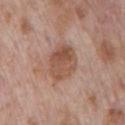Findings:
* lighting · white-light
* subject · male, aged around 70
* acquisition · ~15 mm crop, total-body skin-cancer survey
* body site · the chest